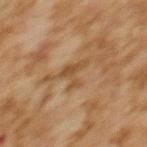Impression: Part of a total-body skin-imaging series; this lesion was reviewed on a skin check and was not flagged for biopsy. Clinical summary: Located on the upper back. Imaged with cross-polarized lighting. A female subject aged 58–62. A region of skin cropped from a whole-body photographic capture, roughly 15 mm wide. Automated tile analysis of the lesion measured an average lesion color of about L≈43 a*≈17 b*≈33 (CIELAB) and a normalized border contrast of about 6.5. The analysis additionally found a border-irregularity index near 8.5/10, internal color variation of about 0.5 on a 0–10 scale, and peripheral color asymmetry of about 0.